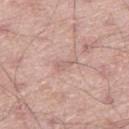Captured during whole-body skin photography for melanoma surveillance; the lesion was not biopsied. Approximately 3 mm at its widest. Cropped from a whole-body photographic skin survey; the tile spans about 15 mm. Automated image analysis of the tile measured an area of roughly 2.5 mm², a shape eccentricity near 0.9, and a shape-asymmetry score of about 0.45 (0 = symmetric). The software also gave a mean CIELAB color near L≈62 a*≈19 b*≈23, about 7 CIELAB-L* units darker than the surrounding skin, and a lesion-to-skin contrast of about 4.5 (normalized; higher = more distinct). The software also gave a lesion-detection confidence of about 75/100. This is a white-light tile. A male patient, about 60 years old. From the right thigh.